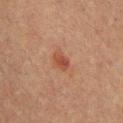Q: Illumination type?
A: cross-polarized illumination
Q: Who is the patient?
A: male, aged around 40
Q: Lesion size?
A: ≈2.5 mm
Q: What is the anatomic site?
A: the chest
Q: What kind of image is this?
A: 15 mm crop, total-body photography